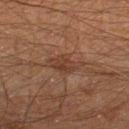Captured during whole-body skin photography for melanoma surveillance; the lesion was not biopsied.
Captured under cross-polarized illumination.
A roughly 15 mm field-of-view crop from a total-body skin photograph.
Measured at roughly 4.5 mm in maximum diameter.
An algorithmic analysis of the crop reported a footprint of about 7 mm², an eccentricity of roughly 0.85, and a symmetry-axis asymmetry near 0.25. And it measured a mean CIELAB color near L≈31 a*≈16 b*≈24, about 6 CIELAB-L* units darker than the surrounding skin, and a normalized border contrast of about 6. The software also gave a lesion-detection confidence of about 100/100.
Located on the right lower leg.
The subject is a male in their mid-40s.